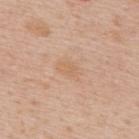Part of a total-body skin-imaging series; this lesion was reviewed on a skin check and was not flagged for biopsy. A male subject, aged 58–62. Located on the upper back. About 3 mm across. This image is a 15 mm lesion crop taken from a total-body photograph.